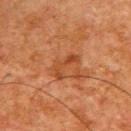The lesion was photographed on a routine skin check and not biopsied; there is no pathology result.
The patient is a male in their mid-60s.
A 15 mm close-up extracted from a 3D total-body photography capture.
This is a cross-polarized tile.
The lesion is located on the upper back.